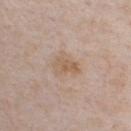Part of a total-body skin-imaging series; this lesion was reviewed on a skin check and was not flagged for biopsy.
Imaged with white-light lighting.
The lesion is located on the chest.
A male subject aged 48–52.
A close-up tile cropped from a whole-body skin photograph, about 15 mm across.